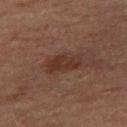| field | value |
|---|---|
| notes | no biopsy performed (imaged during a skin exam) |
| automated lesion analysis | a shape eccentricity near 0.8 and a shape-asymmetry score of about 0.2 (0 = symmetric) |
| site | the right thigh |
| patient | male, aged around 70 |
| image | total-body-photography crop, ~15 mm field of view |